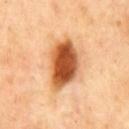Q: Is there a histopathology result?
A: total-body-photography surveillance lesion; no biopsy
Q: How large is the lesion?
A: ~6.5 mm (longest diameter)
Q: What are the patient's age and sex?
A: male, in their 50s
Q: How was this image acquired?
A: ~15 mm tile from a whole-body skin photo
Q: What is the anatomic site?
A: the chest